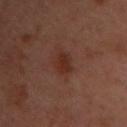Impression:
Imaged during a routine full-body skin examination; the lesion was not biopsied and no histopathology is available.
Context:
The lesion-visualizer software estimated a symmetry-axis asymmetry near 0.25. The software also gave a classifier nevus-likeness of about 55/100. A roughly 15 mm field-of-view crop from a total-body skin photograph. The patient is a female about 55 years old. Approximately 2.5 mm at its widest. The lesion is on the chest. Imaged with cross-polarized lighting.